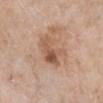<lesion>
  <biopsy_status>not biopsied; imaged during a skin examination</biopsy_status>
  <image>
    <source>total-body photography crop</source>
    <field_of_view_mm>15</field_of_view_mm>
  </image>
  <site>right lower leg</site>
  <lighting>white-light</lighting>
  <lesion_size>
    <long_diameter_mm_approx>4.5</long_diameter_mm_approx>
  </lesion_size>
  <patient>
    <sex>female</sex>
    <age_approx>70</age_approx>
  </patient>
  <automated_metrics>
    <area_mm2_approx>9.0</area_mm2_approx>
    <eccentricity>0.8</eccentricity>
    <shape_asymmetry>0.35</shape_asymmetry>
    <color_variation_0_10>7.0</color_variation_0_10>
    <peripheral_color_asymmetry>2.5</peripheral_color_asymmetry>
  </automated_metrics>
</lesion>